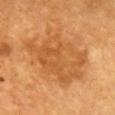No biopsy was performed on this lesion — it was imaged during a full skin examination and was not determined to be concerning.
A region of skin cropped from a whole-body photographic capture, roughly 15 mm wide.
A patient aged around 70.
The lesion's longest dimension is about 9 mm.
This is a cross-polarized tile.
From the chest.